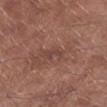| feature | finding |
|---|---|
| workup | no biopsy performed (imaged during a skin exam) |
| subject | male, aged approximately 55 |
| site | the left lower leg |
| image source | total-body-photography crop, ~15 mm field of view |